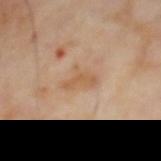notes = no biopsy performed (imaged during a skin exam)
tile lighting = cross-polarized illumination
acquisition = ~15 mm crop, total-body skin-cancer survey
size = ≈3.5 mm
patient = male, about 65 years old
automated lesion analysis = an area of roughly 6 mm², a shape eccentricity near 0.75, and a symmetry-axis asymmetry near 0.45; a classifier nevus-likeness of about 0/100 and lesion-presence confidence of about 100/100